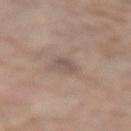Imaged during a routine full-body skin examination; the lesion was not biopsied and no histopathology is available. This image is a 15 mm lesion crop taken from a total-body photograph. Longest diameter approximately 2.5 mm. The tile uses white-light illumination. The lesion is on the lower back. A male patient, aged approximately 80.